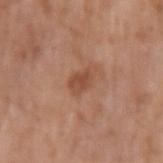| key | value |
|---|---|
| workup | total-body-photography surveillance lesion; no biopsy |
| subject | male, approximately 80 years of age |
| image source | 15 mm crop, total-body photography |
| illumination | white-light |
| location | the left upper arm |